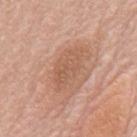Impression:
This lesion was catalogued during total-body skin photography and was not selected for biopsy.
Acquisition and patient details:
A 15 mm close-up tile from a total-body photography series done for melanoma screening. Located on the mid back. Automated tile analysis of the lesion measured an area of roughly 9.5 mm² and an outline eccentricity of about 0.9 (0 = round, 1 = elongated). The analysis additionally found a lesion–skin lightness drop of about 8. The software also gave a within-lesion color-variation index near 3/10. The lesion's longest dimension is about 5.5 mm. A male subject, aged approximately 80.